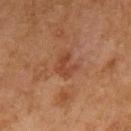{"patient": {"sex": "female", "age_approx": 60}, "site": "arm", "image": {"source": "total-body photography crop", "field_of_view_mm": 15}}